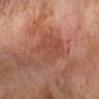| key | value |
|---|---|
| biopsy status | catalogued during a skin exam; not biopsied |
| subject | male, approximately 70 years of age |
| image | 15 mm crop, total-body photography |
| site | the left forearm |